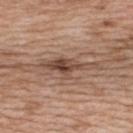The lesion was tiled from a total-body skin photograph and was not biopsied.